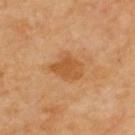biopsy status=no biopsy performed (imaged during a skin exam)
subject=male, aged approximately 70
image source=~15 mm tile from a whole-body skin photo
site=the back